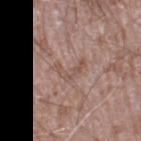Clinical impression: The lesion was tiled from a total-body skin photograph and was not biopsied. Acquisition and patient details: Cropped from a whole-body photographic skin survey; the tile spans about 15 mm. The lesion's longest dimension is about 3.5 mm. The total-body-photography lesion software estimated a lesion area of about 4 mm², an outline eccentricity of about 0.8 (0 = round, 1 = elongated), and a symmetry-axis asymmetry near 0.75. The software also gave a mean CIELAB color near L≈52 a*≈18 b*≈25, roughly 7 lightness units darker than nearby skin, and a lesion-to-skin contrast of about 5.5 (normalized; higher = more distinct). The analysis additionally found internal color variation of about 0 on a 0–10 scale and radial color variation of about 0. A male subject aged around 60. Located on the leg.